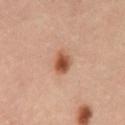Case summary:
– follow-up — total-body-photography surveillance lesion; no biopsy
– site — the abdomen
– patient — male, roughly 40 years of age
– TBP lesion metrics — a lesion area of about 5 mm², an eccentricity of roughly 0.6, and two-axis asymmetry of about 0.2; a lesion color around L≈41 a*≈20 b*≈28 in CIELAB, a lesion–skin lightness drop of about 12, and a normalized lesion–skin contrast near 10
– lighting — cross-polarized
– image — 15 mm crop, total-body photography